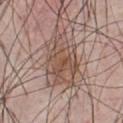The lesion was tiled from a total-body skin photograph and was not biopsied. From the chest. A male patient about 40 years old. Imaged with white-light lighting. A 15 mm close-up tile from a total-body photography series done for melanoma screening.